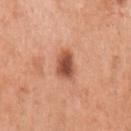Imaged during a routine full-body skin examination; the lesion was not biopsied and no histopathology is available.
The patient is a female aged approximately 65.
Cropped from a total-body skin-imaging series; the visible field is about 15 mm.
Automated tile analysis of the lesion measured a footprint of about 7 mm², an outline eccentricity of about 0.7 (0 = round, 1 = elongated), and a shape-asymmetry score of about 0.25 (0 = symmetric). The analysis additionally found a border-irregularity rating of about 2.5/10 and a within-lesion color-variation index near 4.5/10. It also reported an automated nevus-likeness rating near 95 out of 100 and a detector confidence of about 100 out of 100 that the crop contains a lesion.
The lesion is located on the left upper arm.
Approximately 3.5 mm at its widest.